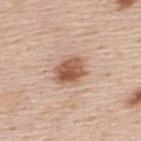  biopsy_status: not biopsied; imaged during a skin examination